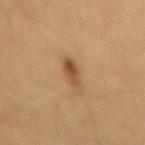Part of a total-body skin-imaging series; this lesion was reviewed on a skin check and was not flagged for biopsy. The lesion is on the mid back. This is a cross-polarized tile. The subject is a male aged around 65. A 15 mm close-up tile from a total-body photography series done for melanoma screening.